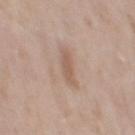notes = total-body-photography surveillance lesion; no biopsy | acquisition = ~15 mm crop, total-body skin-cancer survey | illumination = white-light illumination | lesion diameter = ≈3.5 mm | automated metrics = an area of roughly 4.5 mm², a shape eccentricity near 0.9, and two-axis asymmetry of about 0.25; radial color variation of about 0.5 | location = the mid back | subject = male, in their mid-50s.